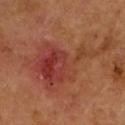Assessment: Captured during whole-body skin photography for melanoma surveillance; the lesion was not biopsied. Context: On the left forearm. Cropped from a whole-body photographic skin survey; the tile spans about 15 mm. Automated image analysis of the tile measured about 9 CIELAB-L* units darker than the surrounding skin and a normalized lesion–skin contrast near 7. And it measured a nevus-likeness score of about 10/100 and lesion-presence confidence of about 100/100. The tile uses cross-polarized illumination. Measured at roughly 11 mm in maximum diameter. The patient is a female aged around 45.